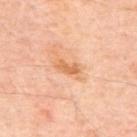Background:
Approximately 3.5 mm at its widest. A male patient, in their mid-60s. A 15 mm close-up tile from a total-body photography series done for melanoma screening. The lesion is on the mid back.